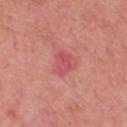biopsy status: catalogued during a skin exam; not biopsied | lesion size: ≈2.5 mm | image-analysis metrics: an eccentricity of roughly 0.55 and a shape-asymmetry score of about 0.35 (0 = symmetric); a border-irregularity rating of about 3.5/10, a within-lesion color-variation index near 3/10, and peripheral color asymmetry of about 1; a nevus-likeness score of about 0/100 and a lesion-detection confidence of about 100/100 | tile lighting: white-light | image source: ~15 mm crop, total-body skin-cancer survey | anatomic site: the head or neck | patient: female, aged 43 to 47.